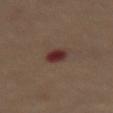Recorded during total-body skin imaging; not selected for excision or biopsy.
A female subject, roughly 65 years of age.
From the abdomen.
A roughly 15 mm field-of-view crop from a total-body skin photograph.